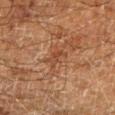The lesion was tiled from a total-body skin photograph and was not biopsied. Approximately 2.5 mm at its widest. The lesion is on the leg. A 15 mm close-up tile from a total-body photography series done for melanoma screening. The subject is a male roughly 60 years of age. Automated image analysis of the tile measured an eccentricity of roughly 0.85. The software also gave a nevus-likeness score of about 0/100. Imaged with cross-polarized lighting.